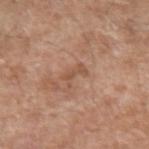A male patient, aged approximately 60.
A 15 mm crop from a total-body photograph taken for skin-cancer surveillance.
From the left upper arm.